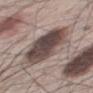biopsy status — catalogued during a skin exam; not biopsied | acquisition — 15 mm crop, total-body photography | patient — male, aged 63–67 | site — the mid back.